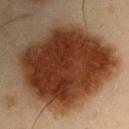biopsy status: catalogued during a skin exam; not biopsied | tile lighting: cross-polarized | automated lesion analysis: a lesion color around L≈28 a*≈19 b*≈26 in CIELAB; a border-irregularity rating of about 1.5/10; a nevus-likeness score of about 100/100 and a lesion-detection confidence of about 100/100 | anatomic site: the left upper arm | acquisition: ~15 mm tile from a whole-body skin photo | lesion size: ≈11 mm | patient: male, roughly 55 years of age.